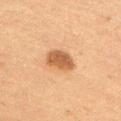Clinical impression:
Imaged during a routine full-body skin examination; the lesion was not biopsied and no histopathology is available.
Acquisition and patient details:
About 3.5 mm across. A close-up tile cropped from a whole-body skin photograph, about 15 mm across. On the right thigh. A male subject, in their mid- to late 70s. Imaged with cross-polarized lighting.